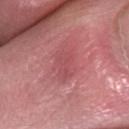From the right lower leg.
A female subject aged 63 to 67.
Measured at roughly 3.5 mm in maximum diameter.
This image is a 15 mm lesion crop taken from a total-body photograph.